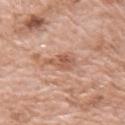This is a white-light tile.
The subject is a female aged 73 to 77.
The lesion is located on the right upper arm.
A 15 mm crop from a total-body photograph taken for skin-cancer surveillance.
The recorded lesion diameter is about 4 mm.